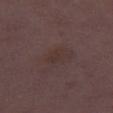notes — no biopsy performed (imaged during a skin exam); site — the leg; patient — female, aged 28–32; image — 15 mm crop, total-body photography.